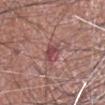biopsy status=no biopsy performed (imaged during a skin exam) | patient=male, in their mid-60s | automated metrics=a shape eccentricity near 0.7 and two-axis asymmetry of about 0.25; a lesion color around L≈47 a*≈26 b*≈20 in CIELAB, roughly 9 lightness units darker than nearby skin, and a normalized lesion–skin contrast near 7.5; a classifier nevus-likeness of about 0/100 and a lesion-detection confidence of about 100/100 | acquisition=15 mm crop, total-body photography | location=the head or neck | lesion size=≈3 mm | tile lighting=white-light illumination.